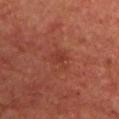No biopsy was performed on this lesion — it was imaged during a full skin examination and was not determined to be concerning. Located on the chest. The lesion-visualizer software estimated a lesion area of about 4 mm² and two-axis asymmetry of about 0.4. The software also gave a classifier nevus-likeness of about 0/100 and a detector confidence of about 100 out of 100 that the crop contains a lesion. The recorded lesion diameter is about 3 mm. Captured under cross-polarized illumination. A roughly 15 mm field-of-view crop from a total-body skin photograph. A male subject, aged around 65.